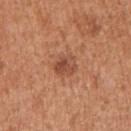Recorded during total-body skin imaging; not selected for excision or biopsy.
A 15 mm close-up tile from a total-body photography series done for melanoma screening.
Automated image analysis of the tile measured a lesion color around L≈49 a*≈25 b*≈32 in CIELAB, a lesion–skin lightness drop of about 9, and a normalized border contrast of about 7. The software also gave a within-lesion color-variation index near 5/10. And it measured a nevus-likeness score of about 45/100.
Measured at roughly 3 mm in maximum diameter.
A female patient aged approximately 40.
Located on the right upper arm.